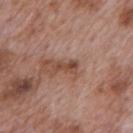- workup · no biopsy performed (imaged during a skin exam)
- site · the back
- imaging modality · ~15 mm crop, total-body skin-cancer survey
- lighting · white-light illumination
- subject · male, aged around 70
- lesion size · ~2.5 mm (longest diameter)